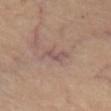Q: Was this lesion biopsied?
A: no biopsy performed (imaged during a skin exam)
Q: Illumination type?
A: cross-polarized illumination
Q: Where on the body is the lesion?
A: the abdomen
Q: Lesion size?
A: ≈3.5 mm
Q: What did automated image analysis measure?
A: an average lesion color of about L≈53 a*≈19 b*≈21 (CIELAB), a lesion–skin lightness drop of about 7, and a lesion-to-skin contrast of about 6 (normalized; higher = more distinct); a color-variation rating of about 0/10; an automated nevus-likeness rating near 0 out of 100
Q: How was this image acquired?
A: ~15 mm tile from a whole-body skin photo